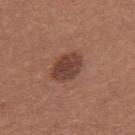workup: catalogued during a skin exam; not biopsied
patient: female, aged approximately 25
image source: total-body-photography crop, ~15 mm field of view
location: the back